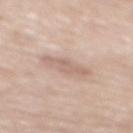Clinical impression: Captured during whole-body skin photography for melanoma surveillance; the lesion was not biopsied. Background: The total-body-photography lesion software estimated a border-irregularity rating of about 2.5/10. From the mid back. Cropped from a total-body skin-imaging series; the visible field is about 15 mm. A male subject aged around 70.